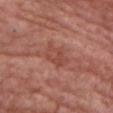Assessment: Imaged during a routine full-body skin examination; the lesion was not biopsied and no histopathology is available. Background: A female subject aged approximately 80. Automated tile analysis of the lesion measured a detector confidence of about 100 out of 100 that the crop contains a lesion. The recorded lesion diameter is about 3.5 mm. The lesion is on the chest. The tile uses white-light illumination. A lesion tile, about 15 mm wide, cut from a 3D total-body photograph.